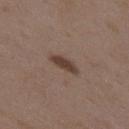Q: Is there a histopathology result?
A: imaged on a skin check; not biopsied
Q: Patient demographics?
A: female, aged 33 to 37
Q: What is the imaging modality?
A: 15 mm crop, total-body photography
Q: What is the anatomic site?
A: the upper back
Q: Illumination type?
A: white-light